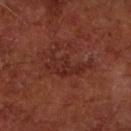Impression:
This lesion was catalogued during total-body skin photography and was not selected for biopsy.
Image and clinical context:
Located on the arm. This is a cross-polarized tile. Automated tile analysis of the lesion measured a border-irregularity rating of about 8.5/10 and a within-lesion color-variation index near 2.5/10. Approximately 5.5 mm at its widest. A 15 mm crop from a total-body photograph taken for skin-cancer surveillance. A male subject aged 63 to 67.